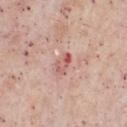Clinical impression:
No biopsy was performed on this lesion — it was imaged during a full skin examination and was not determined to be concerning.
Clinical summary:
A region of skin cropped from a whole-body photographic capture, roughly 15 mm wide. A male patient aged 53–57. On the chest.